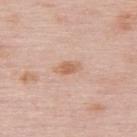{
  "biopsy_status": "not biopsied; imaged during a skin examination",
  "site": "upper back",
  "image": {
    "source": "total-body photography crop",
    "field_of_view_mm": 15
  },
  "patient": {
    "sex": "female",
    "age_approx": 65
  }
}